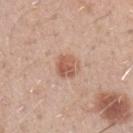  biopsy_status: not biopsied; imaged during a skin examination
  image:
    source: total-body photography crop
    field_of_view_mm: 15
  patient:
    sex: male
    age_approx: 30
  lesion_size:
    long_diameter_mm_approx: 3.0
  site: arm
  lighting: white-light